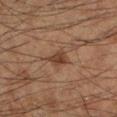Notes:
• image source: ~15 mm tile from a whole-body skin photo
• patient: male, aged approximately 60
• body site: the left lower leg